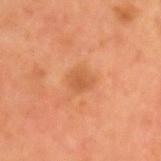follow-up — no biopsy performed (imaged during a skin exam) | image source — 15 mm crop, total-body photography | location — the head or neck | size — ≈3 mm | patient — male, about 55 years old | lighting — cross-polarized illumination.